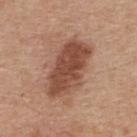Assessment:
Captured during whole-body skin photography for melanoma surveillance; the lesion was not biopsied.
Acquisition and patient details:
From the upper back. A male patient, aged around 65. This is a white-light tile. Measured at roughly 7 mm in maximum diameter. Automated image analysis of the tile measured two-axis asymmetry of about 0.2. And it measured a border-irregularity rating of about 2.5/10, internal color variation of about 4 on a 0–10 scale, and a peripheral color-asymmetry measure near 1.5. The software also gave a classifier nevus-likeness of about 40/100. Cropped from a total-body skin-imaging series; the visible field is about 15 mm.